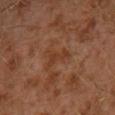Clinical impression: No biopsy was performed on this lesion — it was imaged during a full skin examination and was not determined to be concerning. Acquisition and patient details: From the left leg. The total-body-photography lesion software estimated a lesion area of about 4.5 mm², a shape eccentricity near 0.8, and a shape-asymmetry score of about 0.45 (0 = symmetric). And it measured a classifier nevus-likeness of about 0/100 and lesion-presence confidence of about 100/100. Cropped from a whole-body photographic skin survey; the tile spans about 15 mm. A male patient, aged 28 to 32. The tile uses cross-polarized illumination. About 3 mm across.